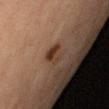The lesion was photographed on a routine skin check and not biopsied; there is no pathology result. The patient is a female aged approximately 50. An algorithmic analysis of the crop reported a footprint of about 4.5 mm² and an eccentricity of roughly 0.8. It also reported a classifier nevus-likeness of about 85/100. On the right upper arm. Captured under cross-polarized illumination. A region of skin cropped from a whole-body photographic capture, roughly 15 mm wide.